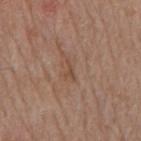biopsy status: no biopsy performed (imaged during a skin exam)
subject: male, about 80 years old
anatomic site: the mid back
size: ~2.5 mm (longest diameter)
lighting: white-light
acquisition: ~15 mm tile from a whole-body skin photo